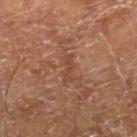Context:
A male patient, aged 68 to 72. On the right lower leg. The lesion's longest dimension is about 3 mm. A 15 mm close-up tile from a total-body photography series done for melanoma screening.